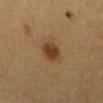Impression: No biopsy was performed on this lesion — it was imaged during a full skin examination and was not determined to be concerning. Clinical summary: This image is a 15 mm lesion crop taken from a total-body photograph. Automated tile analysis of the lesion measured border irregularity of about 1.5 on a 0–10 scale, a color-variation rating of about 2.5/10, and peripheral color asymmetry of about 0.5. The software also gave a nevus-likeness score of about 100/100 and lesion-presence confidence of about 100/100. The subject is a female aged 53 to 57. This is a cross-polarized tile. Located on the abdomen. About 3 mm across.